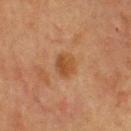A male patient aged around 60.
Measured at roughly 2.5 mm in maximum diameter.
The lesion is on the upper back.
Captured under cross-polarized illumination.
A region of skin cropped from a whole-body photographic capture, roughly 15 mm wide.